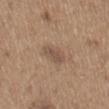This lesion was catalogued during total-body skin photography and was not selected for biopsy. From the back. A male patient, about 65 years old. A close-up tile cropped from a whole-body skin photograph, about 15 mm across. Automated image analysis of the tile measured a border-irregularity rating of about 2/10 and a peripheral color-asymmetry measure near 0.5.